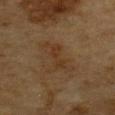workup = catalogued during a skin exam; not biopsied
lighting = cross-polarized
patient = male, aged approximately 85 or older
lesion diameter = ~4.5 mm (longest diameter)
location = the chest
image = ~15 mm crop, total-body skin-cancer survey